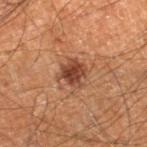lighting: cross-polarized; lesion size: ~3 mm (longest diameter); location: the right lower leg; acquisition: ~15 mm tile from a whole-body skin photo; patient: male, in their 60s.